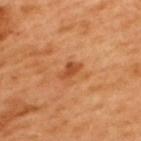notes=catalogued during a skin exam; not biopsied | subject=male, aged 48–52 | image source=~15 mm tile from a whole-body skin photo | body site=the upper back | diameter=~2.5 mm (longest diameter).